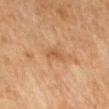{"biopsy_status": "not biopsied; imaged during a skin examination", "patient": {"sex": "female", "age_approx": 60}, "image": {"source": "total-body photography crop", "field_of_view_mm": 15}, "site": "back"}